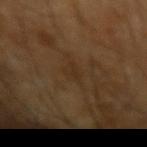No biopsy was performed on this lesion — it was imaged during a full skin examination and was not determined to be concerning.
Automated image analysis of the tile measured border irregularity of about 4.5 on a 0–10 scale, a color-variation rating of about 0.5/10, and a peripheral color-asymmetry measure near 0.5.
Imaged with cross-polarized lighting.
A male patient, about 65 years old.
A 15 mm close-up extracted from a 3D total-body photography capture.
Approximately 3.5 mm at its widest.